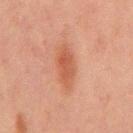Notes:
– follow-up · catalogued during a skin exam; not biopsied
– illumination · cross-polarized
– patient · male, roughly 65 years of age
– image · total-body-photography crop, ~15 mm field of view
– diameter · about 4.5 mm
– anatomic site · the abdomen